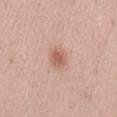follow-up = no biopsy performed (imaged during a skin exam) | image = 15 mm crop, total-body photography | tile lighting = white-light | site = the back | TBP lesion metrics = a mean CIELAB color near L≈60 a*≈21 b*≈29, about 10 CIELAB-L* units darker than the surrounding skin, and a normalized lesion–skin contrast near 7; an automated nevus-likeness rating near 85 out of 100 and lesion-presence confidence of about 100/100 | lesion diameter = about 2.5 mm | subject = male, aged 53 to 57.